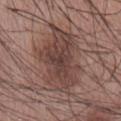Findings:
• biopsy status: no biopsy performed (imaged during a skin exam)
• automated lesion analysis: a nevus-likeness score of about 60/100
• tile lighting: white-light
• imaging modality: total-body-photography crop, ~15 mm field of view
• patient: male, aged 53–57
• diameter: about 9 mm
• site: the abdomen